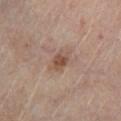Assessment:
Part of a total-body skin-imaging series; this lesion was reviewed on a skin check and was not flagged for biopsy.
Clinical summary:
An algorithmic analysis of the crop reported an area of roughly 4.5 mm² and a symmetry-axis asymmetry near 0.25. The analysis additionally found a classifier nevus-likeness of about 50/100 and a lesion-detection confidence of about 100/100. Cropped from a whole-body photographic skin survey; the tile spans about 15 mm. A male patient aged 58–62. Located on the left lower leg.